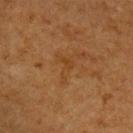Imaged during a routine full-body skin examination; the lesion was not biopsied and no histopathology is available. Cropped from a total-body skin-imaging series; the visible field is about 15 mm. On the chest. Measured at roughly 3.5 mm in maximum diameter. A male subject, about 65 years old.